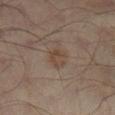Assessment: No biopsy was performed on this lesion — it was imaged during a full skin examination and was not determined to be concerning. Background: A male patient, aged around 65. The lesion is on the leg. This is a cross-polarized tile. Measured at roughly 3 mm in maximum diameter. A close-up tile cropped from a whole-body skin photograph, about 15 mm across. Automated tile analysis of the lesion measured an outline eccentricity of about 0.7 (0 = round, 1 = elongated) and a shape-asymmetry score of about 0.25 (0 = symmetric). The software also gave an average lesion color of about L≈40 a*≈14 b*≈24 (CIELAB). The analysis additionally found lesion-presence confidence of about 100/100.